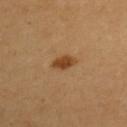Q: Was a biopsy performed?
A: no biopsy performed (imaged during a skin exam)
Q: What kind of image is this?
A: 15 mm crop, total-body photography
Q: What are the patient's age and sex?
A: female, aged approximately 60
Q: Where on the body is the lesion?
A: the left upper arm
Q: What is the lesion's diameter?
A: ~3.5 mm (longest diameter)